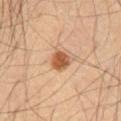Notes:
* biopsy status · no biopsy performed (imaged during a skin exam)
* anatomic site · the abdomen
* subject · male, about 55 years old
* acquisition · total-body-photography crop, ~15 mm field of view